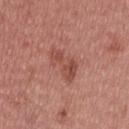Clinical impression:
The lesion was photographed on a routine skin check and not biopsied; there is no pathology result.
Background:
This image is a 15 mm lesion crop taken from a total-body photograph. About 4.5 mm across. A male subject, aged 28–32. This is a white-light tile. Automated image analysis of the tile measured two-axis asymmetry of about 0.5. The software also gave a lesion–skin lightness drop of about 9 and a normalized lesion–skin contrast near 6.5. The software also gave a border-irregularity rating of about 6/10, a color-variation rating of about 3.5/10, and peripheral color asymmetry of about 1.5.